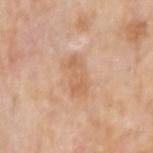workup: imaged on a skin check; not biopsied
subject: male, roughly 75 years of age
site: the right upper arm
automated lesion analysis: a footprint of about 9 mm² and an eccentricity of roughly 0.85; a within-lesion color-variation index near 2/10
imaging modality: total-body-photography crop, ~15 mm field of view
illumination: white-light